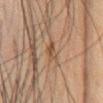workup = catalogued during a skin exam; not biopsied | subject = male, roughly 65 years of age | automated metrics = a lesion-detection confidence of about 90/100 | anatomic site = the chest | image source = total-body-photography crop, ~15 mm field of view.